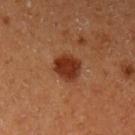<record>
  <biopsy_status>not biopsied; imaged during a skin examination</biopsy_status>
  <lighting>cross-polarized</lighting>
  <patient>
    <sex>female</sex>
    <age_approx>50</age_approx>
  </patient>
  <image>
    <source>total-body photography crop</source>
    <field_of_view_mm>15</field_of_view_mm>
  </image>
  <site>left upper arm</site>
</record>